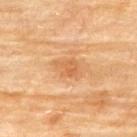Notes:
– notes — imaged on a skin check; not biopsied
– lesion size — ~3.5 mm (longest diameter)
– site — the upper back
– automated lesion analysis — a footprint of about 5.5 mm² and an eccentricity of roughly 0.75; an average lesion color of about L≈54 a*≈21 b*≈37 (CIELAB), a lesion–skin lightness drop of about 7, and a normalized border contrast of about 5.5
– subject — female, aged 78–82
– illumination — cross-polarized illumination
– image source — total-body-photography crop, ~15 mm field of view